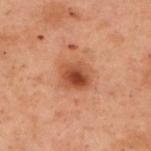The lesion was tiled from a total-body skin photograph and was not biopsied. From the back. About 3 mm across. A female patient, in their mid- to late 50s. A 15 mm close-up extracted from a 3D total-body photography capture.